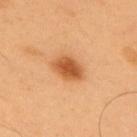notes = imaged on a skin check; not biopsied.